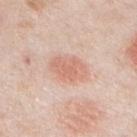Clinical impression:
The lesion was photographed on a routine skin check and not biopsied; there is no pathology result.
Clinical summary:
Automated tile analysis of the lesion measured an average lesion color of about L≈67 a*≈23 b*≈29 (CIELAB) and a lesion–skin lightness drop of about 9. And it measured a border-irregularity rating of about 2/10, a within-lesion color-variation index near 2.5/10, and a peripheral color-asymmetry measure near 1. The software also gave a nevus-likeness score of about 100/100. This image is a 15 mm lesion crop taken from a total-body photograph. A male subject, aged 48 to 52. From the chest.